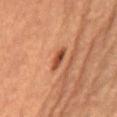The lesion was tiled from a total-body skin photograph and was not biopsied.
The patient is a female approximately 70 years of age.
The lesion-visualizer software estimated a lesion area of about 3.5 mm² and two-axis asymmetry of about 0.25. And it measured roughly 12 lightness units darker than nearby skin and a normalized lesion–skin contrast near 9.5. And it measured a border-irregularity rating of about 3/10 and peripheral color asymmetry of about 1. The software also gave a classifier nevus-likeness of about 10/100 and lesion-presence confidence of about 100/100.
About 3.5 mm across.
The lesion is on the abdomen.
Imaged with cross-polarized lighting.
A 15 mm close-up tile from a total-body photography series done for melanoma screening.